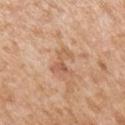Q: Was a biopsy performed?
A: catalogued during a skin exam; not biopsied
Q: Who is the patient?
A: male, aged approximately 65
Q: Lesion location?
A: the right upper arm
Q: What kind of image is this?
A: total-body-photography crop, ~15 mm field of view